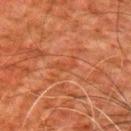workup: no biopsy performed (imaged during a skin exam)
subject: male, aged 78–82
image: ~15 mm tile from a whole-body skin photo
anatomic site: the right upper arm
size: about 3 mm
tile lighting: cross-polarized illumination